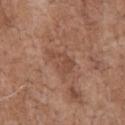Captured during whole-body skin photography for melanoma surveillance; the lesion was not biopsied.
On the chest.
A 15 mm crop from a total-body photograph taken for skin-cancer surveillance.
The subject is a male about 70 years old.
Captured under white-light illumination.